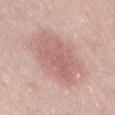No biopsy was performed on this lesion — it was imaged during a full skin examination and was not determined to be concerning. The lesion's longest dimension is about 7 mm. A 15 mm close-up tile from a total-body photography series done for melanoma screening. The lesion-visualizer software estimated a shape eccentricity near 0.7 and a symmetry-axis asymmetry near 0.15. The software also gave lesion-presence confidence of about 100/100. From the lower back. The subject is a female roughly 30 years of age.